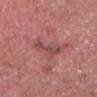Impression:
Recorded during total-body skin imaging; not selected for excision or biopsy.
Background:
On the head or neck. A 15 mm close-up tile from a total-body photography series done for melanoma screening. A male patient, roughly 75 years of age. Captured under white-light illumination. Longest diameter approximately 4 mm.